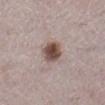This lesion was catalogued during total-body skin photography and was not selected for biopsy. Cropped from a total-body skin-imaging series; the visible field is about 15 mm. From the left lower leg. A female patient aged around 50. This is a white-light tile. Approximately 3 mm at its widest.